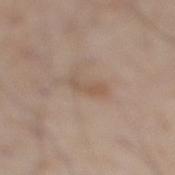Findings:
– illumination: white-light
– lesion size: ≈3 mm
– site: the right lower leg
– subject: male, aged approximately 60
– image source: total-body-photography crop, ~15 mm field of view